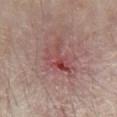Recorded during total-body skin imaging; not selected for excision or biopsy. A 15 mm close-up extracted from a 3D total-body photography capture. The lesion is on the chest. Automated image analysis of the tile measured a nevus-likeness score of about 0/100. The lesion's longest dimension is about 4.5 mm. The subject is a male about 80 years old. This is a white-light tile.